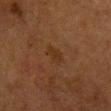  biopsy_status: not biopsied; imaged during a skin examination
  lesion_size:
    long_diameter_mm_approx: 2.5
  image:
    source: total-body photography crop
    field_of_view_mm: 15
  lighting: cross-polarized
  site: chest
  patient:
    sex: female
    age_approx: 55